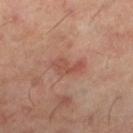Recorded during total-body skin imaging; not selected for excision or biopsy. Automated tile analysis of the lesion measured a shape eccentricity near 0.85 and a symmetry-axis asymmetry near 0.4. It also reported a mean CIELAB color near L≈46 a*≈23 b*≈27, a lesion–skin lightness drop of about 7, and a lesion-to-skin contrast of about 6 (normalized; higher = more distinct). The software also gave a color-variation rating of about 1.5/10 and peripheral color asymmetry of about 0.5. And it measured a classifier nevus-likeness of about 5/100. A male patient aged around 60. The lesion's longest dimension is about 3.5 mm. A close-up tile cropped from a whole-body skin photograph, about 15 mm across. Imaged with cross-polarized lighting. On the leg.